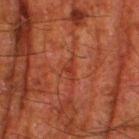Assessment:
The lesion was tiled from a total-body skin photograph and was not biopsied.
Context:
Longest diameter approximately 2.5 mm. This is a cross-polarized tile. Located on the right thigh. A close-up tile cropped from a whole-body skin photograph, about 15 mm across. A male patient aged 78–82.